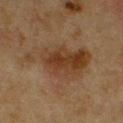notes = no biopsy performed (imaged during a skin exam) | diameter = about 8.5 mm | image = ~15 mm crop, total-body skin-cancer survey | lighting = cross-polarized | anatomic site = the chest | patient = male, aged 73 to 77.